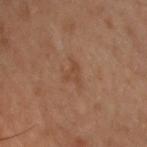Q: Is there a histopathology result?
A: no biopsy performed (imaged during a skin exam)